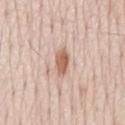The lesion was tiled from a total-body skin photograph and was not biopsied. A region of skin cropped from a whole-body photographic capture, roughly 15 mm wide. The lesion is located on the mid back. Captured under white-light illumination. A male subject, roughly 80 years of age. The recorded lesion diameter is about 3.5 mm. Automated image analysis of the tile measured an area of roughly 5 mm² and two-axis asymmetry of about 0.2. And it measured an automated nevus-likeness rating near 100 out of 100 and a detector confidence of about 100 out of 100 that the crop contains a lesion.